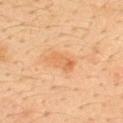The lesion was tiled from a total-body skin photograph and was not biopsied. A male subject, approximately 35 years of age. The lesion is located on the upper back. A region of skin cropped from a whole-body photographic capture, roughly 15 mm wide.